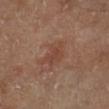The lesion was photographed on a routine skin check and not biopsied; there is no pathology result. This is a cross-polarized tile. Automated image analysis of the tile measured a border-irregularity rating of about 3.5/10, a within-lesion color-variation index near 2/10, and radial color variation of about 1. The software also gave a classifier nevus-likeness of about 0/100 and a lesion-detection confidence of about 100/100. A region of skin cropped from a whole-body photographic capture, roughly 15 mm wide. Measured at roughly 3.5 mm in maximum diameter. Located on the right lower leg. A male subject approximately 70 years of age.